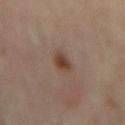Part of a total-body skin-imaging series; this lesion was reviewed on a skin check and was not flagged for biopsy.
The lesion is on the mid back.
A female subject aged approximately 60.
This is a cross-polarized tile.
A lesion tile, about 15 mm wide, cut from a 3D total-body photograph.